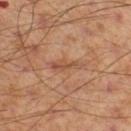biopsy status: catalogued during a skin exam; not biopsied
image: ~15 mm tile from a whole-body skin photo
subject: male, aged 53 to 57
lighting: cross-polarized illumination
TBP lesion metrics: an average lesion color of about L≈52 a*≈23 b*≈32 (CIELAB), a lesion–skin lightness drop of about 7, and a lesion-to-skin contrast of about 5.5 (normalized; higher = more distinct)
site: the left thigh
lesion size: about 3.5 mm